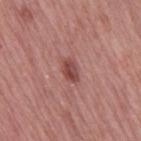A roughly 15 mm field-of-view crop from a total-body skin photograph. Imaged with white-light lighting. The lesion is on the leg. The subject is a female about 65 years old. About 3 mm across.